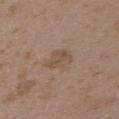Automated tile analysis of the lesion measured a footprint of about 6 mm², a shape eccentricity near 0.75, and a symmetry-axis asymmetry near 0.35. It also reported a lesion-to-skin contrast of about 5.5 (normalized; higher = more distinct). The analysis additionally found a border-irregularity rating of about 3.5/10, a within-lesion color-variation index near 2/10, and peripheral color asymmetry of about 1. And it measured an automated nevus-likeness rating near 0 out of 100 and a detector confidence of about 100 out of 100 that the crop contains a lesion.
A roughly 15 mm field-of-view crop from a total-body skin photograph.
The subject is a female aged approximately 35.
The lesion is located on the upper back.